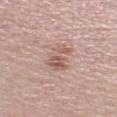Context:
This is a white-light tile. Measured at roughly 4 mm in maximum diameter. A female subject, roughly 65 years of age. The lesion-visualizer software estimated a border-irregularity index near 6/10, a within-lesion color-variation index near 4/10, and radial color variation of about 1.5. The analysis additionally found a classifier nevus-likeness of about 0/100 and a detector confidence of about 100 out of 100 that the crop contains a lesion. A 15 mm close-up extracted from a 3D total-body photography capture. From the left lower leg.